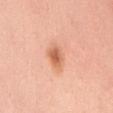Recorded during total-body skin imaging; not selected for excision or biopsy.
Cropped from a whole-body photographic skin survey; the tile spans about 15 mm.
The tile uses white-light illumination.
A female patient about 50 years old.
The lesion's longest dimension is about 3 mm.
The lesion is on the mid back.
An algorithmic analysis of the crop reported a peripheral color-asymmetry measure near 1.5.